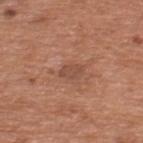Impression: The lesion was tiled from a total-body skin photograph and was not biopsied. Acquisition and patient details: A 15 mm crop from a total-body photograph taken for skin-cancer surveillance. The total-body-photography lesion software estimated a lesion area of about 3.5 mm² and a shape-asymmetry score of about 0.2 (0 = symmetric). It also reported a border-irregularity index near 2/10, a color-variation rating of about 1.5/10, and a peripheral color-asymmetry measure near 0.5. It also reported a classifier nevus-likeness of about 0/100 and lesion-presence confidence of about 100/100. Imaged with white-light lighting. A male subject in their mid-60s. Measured at roughly 2.5 mm in maximum diameter. From the upper back.